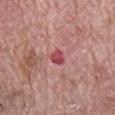{"biopsy_status": "not biopsied; imaged during a skin examination", "site": "mid back", "automated_metrics": {"area_mm2_approx": 3.0, "eccentricity": 0.75, "shape_asymmetry": 0.35, "cielab_L": 49, "cielab_a": 34, "cielab_b": 20, "vs_skin_darker_L": 12.0, "vs_skin_contrast_norm": 8.5}, "lighting": "white-light", "patient": {"sex": "male", "age_approx": 70}, "image": {"source": "total-body photography crop", "field_of_view_mm": 15}}